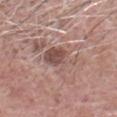Q: Is there a histopathology result?
A: catalogued during a skin exam; not biopsied